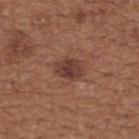Clinical summary:
A 15 mm crop from a total-body photograph taken for skin-cancer surveillance. A female subject approximately 65 years of age. The total-body-photography lesion software estimated a mean CIELAB color near L≈39 a*≈21 b*≈25. The analysis additionally found a border-irregularity index near 2/10, a color-variation rating of about 3.5/10, and a peripheral color-asymmetry measure near 1. The analysis additionally found an automated nevus-likeness rating near 80 out of 100 and a lesion-detection confidence of about 100/100. About 3.5 mm across. On the upper back.